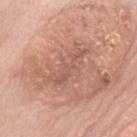No biopsy was performed on this lesion — it was imaged during a full skin examination and was not determined to be concerning. From the mid back. The lesion-visualizer software estimated an area of roughly 7.5 mm² and a shape-asymmetry score of about 0.45 (0 = symmetric). And it measured radial color variation of about 0. This is a white-light tile. A region of skin cropped from a whole-body photographic capture, roughly 15 mm wide. A male subject in their 80s. The recorded lesion diameter is about 5.5 mm.